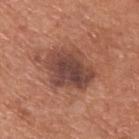Recorded during total-body skin imaging; not selected for excision or biopsy. Approximately 5.5 mm at its widest. An algorithmic analysis of the crop reported a border-irregularity index near 2.5/10, a color-variation rating of about 5.5/10, and peripheral color asymmetry of about 1.5. The lesion is located on the upper back. A roughly 15 mm field-of-view crop from a total-body skin photograph. A male patient, in their mid-60s.